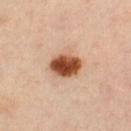automated metrics=an area of roughly 9.5 mm², a shape eccentricity near 0.7, and a symmetry-axis asymmetry near 0.1; a nevus-likeness score of about 100/100 and lesion-presence confidence of about 100/100
anatomic site=the leg
subject=female, aged around 40
image source=~15 mm crop, total-body skin-cancer survey
lesion size=about 4 mm
lighting=cross-polarized illumination